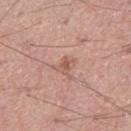– notes — catalogued during a skin exam; not biopsied
– subject — male, aged around 55
– automated lesion analysis — a shape eccentricity near 0.75; an average lesion color of about L≈57 a*≈22 b*≈28 (CIELAB); a border-irregularity rating of about 3.5/10 and a peripheral color-asymmetry measure near 1; an automated nevus-likeness rating near 0 out of 100
– image source — ~15 mm tile from a whole-body skin photo